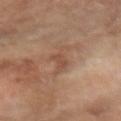Clinical impression:
Recorded during total-body skin imaging; not selected for excision or biopsy.
Background:
From the left forearm. A roughly 15 mm field-of-view crop from a total-body skin photograph. Automated tile analysis of the lesion measured a lesion area of about 5 mm², an outline eccentricity of about 0.95 (0 = round, 1 = elongated), and two-axis asymmetry of about 0.45. The software also gave a border-irregularity rating of about 7/10 and radial color variation of about 0.5. It also reported a classifier nevus-likeness of about 0/100 and lesion-presence confidence of about 75/100. About 4.5 mm across. A female patient, about 70 years old. This is a cross-polarized tile.